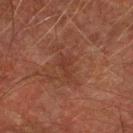Q: Is there a histopathology result?
A: imaged on a skin check; not biopsied
Q: What is the imaging modality?
A: 15 mm crop, total-body photography
Q: Patient demographics?
A: male, aged 73 to 77
Q: Where on the body is the lesion?
A: the arm